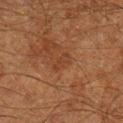Captured during whole-body skin photography for melanoma surveillance; the lesion was not biopsied.
This image is a 15 mm lesion crop taken from a total-body photograph.
Located on the right lower leg.
A male subject aged around 60.